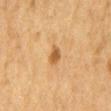workup: no biopsy performed (imaged during a skin exam) | size: ~3 mm (longest diameter) | automated lesion analysis: an area of roughly 3.5 mm² and an eccentricity of roughly 0.85; a mean CIELAB color near L≈50 a*≈19 b*≈38, about 10 CIELAB-L* units darker than the surrounding skin, and a normalized lesion–skin contrast near 8; a classifier nevus-likeness of about 75/100 and lesion-presence confidence of about 100/100 | site: the mid back | tile lighting: cross-polarized | patient: male, aged around 85 or older | image: total-body-photography crop, ~15 mm field of view.